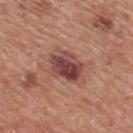workup = catalogued during a skin exam; not biopsied
size = about 4 mm
subject = male, aged 73 to 77
lighting = white-light
image = ~15 mm tile from a whole-body skin photo
body site = the back